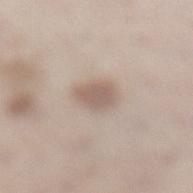diameter — about 3 mm
patient — female, approximately 45 years of age
imaging modality — total-body-photography crop, ~15 mm field of view
body site — the left lower leg
illumination — white-light illumination